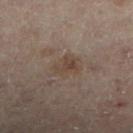The lesion was tiled from a total-body skin photograph and was not biopsied.
A female subject aged around 55.
Located on the left lower leg.
Captured under cross-polarized illumination.
About 3 mm across.
This image is a 15 mm lesion crop taken from a total-body photograph.